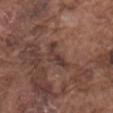No biopsy was performed on this lesion — it was imaged during a full skin examination and was not determined to be concerning. The lesion is on the mid back. A 15 mm close-up extracted from a 3D total-body photography capture. A male subject in their mid-70s. The lesion's longest dimension is about 4.5 mm. Imaged with white-light lighting. The total-body-photography lesion software estimated about 8 CIELAB-L* units darker than the surrounding skin and a normalized border contrast of about 7.5. The analysis additionally found a border-irregularity rating of about 6/10, a color-variation rating of about 3.5/10, and peripheral color asymmetry of about 1.5. And it measured a classifier nevus-likeness of about 5/100 and a lesion-detection confidence of about 55/100.